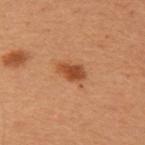The lesion is located on the left upper arm.
The subject is a female about 50 years old.
A roughly 15 mm field-of-view crop from a total-body skin photograph.
The recorded lesion diameter is about 3.5 mm.
Captured under cross-polarized illumination.